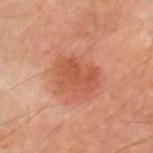{
  "biopsy_status": "not biopsied; imaged during a skin examination",
  "image": {
    "source": "total-body photography crop",
    "field_of_view_mm": 15
  },
  "lighting": "cross-polarized",
  "patient": {
    "sex": "male",
    "age_approx": 70
  },
  "site": "leg",
  "lesion_size": {
    "long_diameter_mm_approx": 5.0
  }
}